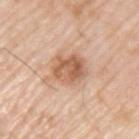Case summary:
– biopsy status — total-body-photography surveillance lesion; no biopsy
– diameter — ≈4 mm
– tile lighting — white-light illumination
– site — the left upper arm
– patient — male, aged 63–67
– image — ~15 mm tile from a whole-body skin photo
– TBP lesion metrics — an area of roughly 11 mm²; a lesion color around L≈60 a*≈21 b*≈33 in CIELAB, roughly 12 lightness units darker than nearby skin, and a lesion-to-skin contrast of about 7.5 (normalized; higher = more distinct); a border-irregularity index near 2.5/10, internal color variation of about 5.5 on a 0–10 scale, and a peripheral color-asymmetry measure near 2